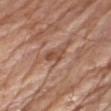Notes:
• biopsy status — catalogued during a skin exam; not biopsied
• diameter — about 3 mm
• illumination — white-light
• patient — male, about 80 years old
• site — the right upper arm
• automated lesion analysis — an area of roughly 4 mm², a shape eccentricity near 0.85, and a shape-asymmetry score of about 0.35 (0 = symmetric); a lesion color around L≈50 a*≈22 b*≈30 in CIELAB and a normalized border contrast of about 6.5
• image — ~15 mm tile from a whole-body skin photo